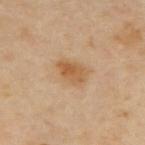The lesion was photographed on a routine skin check and not biopsied; there is no pathology result. The lesion is located on the upper back. About 3.5 mm across. A male subject, aged 63 to 67. A region of skin cropped from a whole-body photographic capture, roughly 15 mm wide.